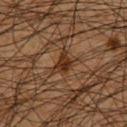Assessment: The lesion was tiled from a total-body skin photograph and was not biopsied. Background: A close-up tile cropped from a whole-body skin photograph, about 15 mm across. Measured at roughly 3 mm in maximum diameter. Automated image analysis of the tile measured a footprint of about 4 mm², an eccentricity of roughly 0.75, and two-axis asymmetry of about 0.45. A male subject, about 65 years old. Imaged with cross-polarized lighting. The lesion is on the front of the torso.